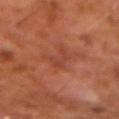An algorithmic analysis of the crop reported a lesion color around L≈40 a*≈27 b*≈30 in CIELAB and a normalized lesion–skin contrast near 4.5. The software also gave a classifier nevus-likeness of about 0/100 and a lesion-detection confidence of about 100/100. Captured under cross-polarized illumination. Cropped from a whole-body photographic skin survey; the tile spans about 15 mm. The lesion is located on the left forearm. Longest diameter approximately 3.5 mm. A male subject about 60 years old.